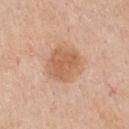This lesion was catalogued during total-body skin photography and was not selected for biopsy.